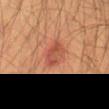  biopsy_status: not biopsied; imaged during a skin examination
  lighting: cross-polarized
  patient:
    sex: male
    age_approx: 65
  site: right thigh
  automated_metrics:
    area_mm2_approx: 6.5
    eccentricity: 0.75
    shape_asymmetry: 0.2
  image:
    source: total-body photography crop
    field_of_view_mm: 15